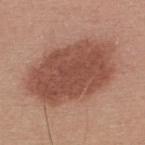notes: total-body-photography surveillance lesion; no biopsy
patient: male, in their 30s
location: the upper back
lighting: white-light
lesion size: ~10 mm (longest diameter)
acquisition: 15 mm crop, total-body photography